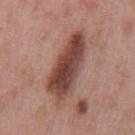This lesion was catalogued during total-body skin photography and was not selected for biopsy.
Located on the mid back.
A roughly 15 mm field-of-view crop from a total-body skin photograph.
The subject is a male approximately 55 years of age.
Measured at roughly 8 mm in maximum diameter.
Automated tile analysis of the lesion measured a mean CIELAB color near L≈44 a*≈22 b*≈24, about 15 CIELAB-L* units darker than the surrounding skin, and a lesion-to-skin contrast of about 11 (normalized; higher = more distinct). The software also gave a border-irregularity rating of about 3/10, internal color variation of about 6 on a 0–10 scale, and a peripheral color-asymmetry measure near 2. The software also gave a nevus-likeness score of about 25/100 and a detector confidence of about 100 out of 100 that the crop contains a lesion.